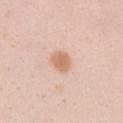The lesion was photographed on a routine skin check and not biopsied; there is no pathology result. The lesion is on the right upper arm. Approximately 3 mm at its widest. The tile uses white-light illumination. A female patient roughly 20 years of age. A roughly 15 mm field-of-view crop from a total-body skin photograph.